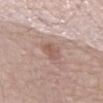| field | value |
|---|---|
| location | the mid back |
| lesion diameter | ~2.5 mm (longest diameter) |
| subject | male, in their mid- to late 80s or older |
| lighting | white-light |
| imaging modality | ~15 mm crop, total-body skin-cancer survey |